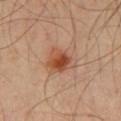Part of a total-body skin-imaging series; this lesion was reviewed on a skin check and was not flagged for biopsy. Automated tile analysis of the lesion measured a mean CIELAB color near L≈52 a*≈23 b*≈32, a lesion–skin lightness drop of about 9, and a normalized lesion–skin contrast near 7. And it measured a nevus-likeness score of about 95/100 and a detector confidence of about 100 out of 100 that the crop contains a lesion. Located on the front of the torso. A 15 mm close-up extracted from a 3D total-body photography capture. The subject is a male roughly 40 years of age. This is a cross-polarized tile. About 4 mm across.